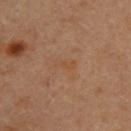Recorded during total-body skin imaging; not selected for excision or biopsy. A female subject roughly 40 years of age. Located on the upper back. Cropped from a whole-body photographic skin survey; the tile spans about 15 mm.